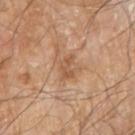The lesion was photographed on a routine skin check and not biopsied; there is no pathology result.
A male subject, approximately 80 years of age.
From the arm.
This is a white-light tile.
Cropped from a total-body skin-imaging series; the visible field is about 15 mm.
The lesion's longest dimension is about 2.5 mm.
An algorithmic analysis of the crop reported a classifier nevus-likeness of about 10/100 and a lesion-detection confidence of about 100/100.